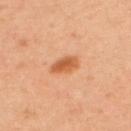Q: Was a biopsy performed?
A: no biopsy performed (imaged during a skin exam)
Q: How was this image acquired?
A: ~15 mm crop, total-body skin-cancer survey
Q: Lesion size?
A: ~3 mm (longest diameter)
Q: Patient demographics?
A: male, about 40 years old
Q: Lesion location?
A: the upper back
Q: What did automated image analysis measure?
A: an area of roughly 5.5 mm² and a shape eccentricity near 0.8; a border-irregularity rating of about 2/10, a color-variation rating of about 3/10, and a peripheral color-asymmetry measure near 1